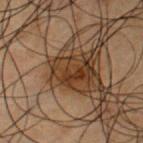Case summary:
– biopsy status: total-body-photography surveillance lesion; no biopsy
– subject: male, aged around 50
– image source: 15 mm crop, total-body photography
– lesion size: ~4.5 mm (longest diameter)
– lighting: cross-polarized
– body site: the chest